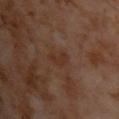workup: catalogued during a skin exam; not biopsied
image source: ~15 mm tile from a whole-body skin photo
patient: male, roughly 60 years of age
site: the chest